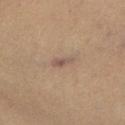Impression: Captured during whole-body skin photography for melanoma surveillance; the lesion was not biopsied. Acquisition and patient details: A male subject, about 60 years old. Cropped from a whole-body photographic skin survey; the tile spans about 15 mm. Imaged with cross-polarized lighting. Located on the right lower leg. Measured at roughly 3 mm in maximum diameter.